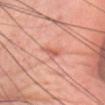workup = total-body-photography surveillance lesion; no biopsy
imaging modality = ~15 mm tile from a whole-body skin photo
patient = female, aged 58 to 62
site = the head or neck
lighting = white-light illumination
diameter = ≈2.5 mm